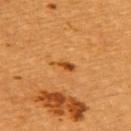Imaged during a routine full-body skin examination; the lesion was not biopsied and no histopathology is available.
A 15 mm crop from a total-body photograph taken for skin-cancer surveillance.
The lesion is located on the back.
Approximately 3 mm at its widest.
The patient is a female roughly 55 years of age.
Imaged with cross-polarized lighting.